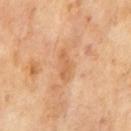automated metrics: a footprint of about 5 mm², an eccentricity of roughly 0.9, and a shape-asymmetry score of about 0.3 (0 = symmetric); border irregularity of about 3.5 on a 0–10 scale and a peripheral color-asymmetry measure near 0.5
size: ≈4 mm
subject: male, roughly 65 years of age
image: total-body-photography crop, ~15 mm field of view
tile lighting: cross-polarized illumination